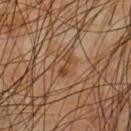Imaged during a routine full-body skin examination; the lesion was not biopsied and no histopathology is available. A male subject, aged approximately 65. From the front of the torso. A 15 mm close-up tile from a total-body photography series done for melanoma screening. Imaged with cross-polarized lighting.